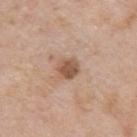Q: Was this lesion biopsied?
A: total-body-photography surveillance lesion; no biopsy
Q: Who is the patient?
A: male, aged 58–62
Q: What lighting was used for the tile?
A: white-light illumination
Q: What is the anatomic site?
A: the left upper arm
Q: How was this image acquired?
A: total-body-photography crop, ~15 mm field of view
Q: What is the lesion's diameter?
A: ≈2.5 mm